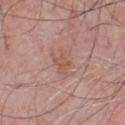Q: Was this lesion biopsied?
A: catalogued during a skin exam; not biopsied
Q: Where on the body is the lesion?
A: the chest
Q: What lighting was used for the tile?
A: white-light illumination
Q: Who is the patient?
A: male, aged around 65
Q: What is the imaging modality?
A: ~15 mm tile from a whole-body skin photo